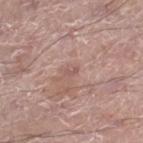Case summary:
– follow-up · no biopsy performed (imaged during a skin exam)
– automated lesion analysis · a mean CIELAB color near L≈54 a*≈21 b*≈22 and roughly 7 lightness units darker than nearby skin; a border-irregularity index near 5.5/10, a color-variation rating of about 0/10, and radial color variation of about 0; a nevus-likeness score of about 0/100
– image source · ~15 mm crop, total-body skin-cancer survey
– subject · male, aged approximately 70
– location · the right lower leg
– illumination · white-light illumination